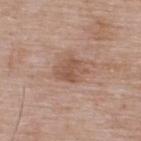{"biopsy_status": "not biopsied; imaged during a skin examination", "lesion_size": {"long_diameter_mm_approx": 3.5}, "site": "upper back", "patient": {"sex": "male", "age_approx": 65}, "image": {"source": "total-body photography crop", "field_of_view_mm": 15}, "lighting": "white-light"}